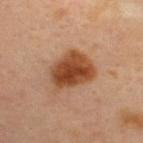  biopsy_status: not biopsied; imaged during a skin examination
  automated_metrics:
    border_irregularity_0_10: 2.0
    color_variation_0_10: 5.0
  lighting: cross-polarized
  image:
    source: total-body photography crop
    field_of_view_mm: 15
  patient:
    sex: male
    age_approx: 35
  site: upper back